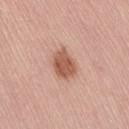The lesion was tiled from a total-body skin photograph and was not biopsied. The subject is a male aged 68 to 72. This image is a 15 mm lesion crop taken from a total-body photograph. On the lower back. About 4 mm across.